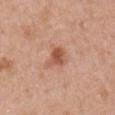No biopsy was performed on this lesion — it was imaged during a full skin examination and was not determined to be concerning. Imaged with white-light lighting. A 15 mm close-up tile from a total-body photography series done for melanoma screening. The lesion is on the right upper arm. The total-body-photography lesion software estimated an average lesion color of about L≈54 a*≈25 b*≈32 (CIELAB), about 11 CIELAB-L* units darker than the surrounding skin, and a lesion-to-skin contrast of about 7.5 (normalized; higher = more distinct). The software also gave a nevus-likeness score of about 55/100 and lesion-presence confidence of about 100/100. The patient is a female aged approximately 50. Approximately 3 mm at its widest.